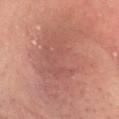Context: A 15 mm crop from a total-body photograph taken for skin-cancer surveillance. Imaged with cross-polarized lighting. The lesion's longest dimension is about 15 mm. A female patient, aged around 30. Automated image analysis of the tile measured a footprint of about 60 mm², a shape eccentricity near 0.95, and two-axis asymmetry of about 0.3. The analysis additionally found a lesion–skin lightness drop of about 7 and a lesion-to-skin contrast of about 5.5 (normalized; higher = more distinct). The analysis additionally found a border-irregularity index near 5.5/10 and a color-variation rating of about 4/10. On the head or neck.